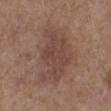No biopsy was performed on this lesion — it was imaged during a full skin examination and was not determined to be concerning.
The tile uses white-light illumination.
A lesion tile, about 15 mm wide, cut from a 3D total-body photograph.
The patient is a female roughly 65 years of age.
The lesion-visualizer software estimated a nevus-likeness score of about 5/100 and a detector confidence of about 100 out of 100 that the crop contains a lesion.
The lesion is located on the left lower leg.
Longest diameter approximately 7 mm.